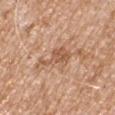Case summary:
- biopsy status: no biopsy performed (imaged during a skin exam)
- image source: ~15 mm tile from a whole-body skin photo
- subject: male, about 65 years old
- diameter: about 4.5 mm
- anatomic site: the right upper arm
- tile lighting: white-light